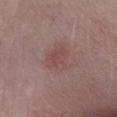No biopsy was performed on this lesion — it was imaged during a full skin examination and was not determined to be concerning. Automated image analysis of the tile measured a lesion area of about 8 mm², a shape eccentricity near 0.65, and a symmetry-axis asymmetry near 0.25. It also reported an average lesion color of about L≈47 a*≈20 b*≈20 (CIELAB), about 6 CIELAB-L* units darker than the surrounding skin, and a lesion-to-skin contrast of about 5 (normalized; higher = more distinct). The analysis additionally found a border-irregularity index near 2.5/10, a within-lesion color-variation index near 2/10, and radial color variation of about 0.5. It also reported a nevus-likeness score of about 60/100 and a lesion-detection confidence of about 100/100. Captured under white-light illumination. A roughly 15 mm field-of-view crop from a total-body skin photograph. A male patient, about 55 years old. Located on the right lower leg.